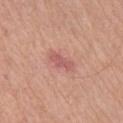Findings:
- workup — total-body-photography surveillance lesion; no biopsy
- subject — male, aged approximately 60
- anatomic site — the right upper arm
- imaging modality — 15 mm crop, total-body photography